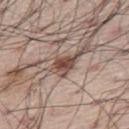No biopsy was performed on this lesion — it was imaged during a full skin examination and was not determined to be concerning. This is a white-light tile. The lesion is on the leg. A male patient, about 70 years old. A roughly 15 mm field-of-view crop from a total-body skin photograph.